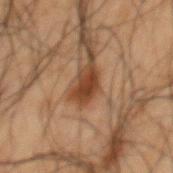notes = no biopsy performed (imaged during a skin exam) | image source = total-body-photography crop, ~15 mm field of view | body site = the mid back | subject = male, aged approximately 50 | lighting = cross-polarized illumination | lesion size = ~2.5 mm (longest diameter).